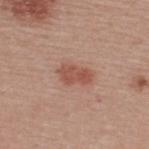Findings:
– biopsy status · total-body-photography surveillance lesion; no biopsy
– location · the upper back
– tile lighting · white-light illumination
– patient · male, about 40 years old
– lesion diameter · ~3.5 mm (longest diameter)
– acquisition · total-body-photography crop, ~15 mm field of view
– TBP lesion metrics · border irregularity of about 3 on a 0–10 scale, a within-lesion color-variation index near 2.5/10, and a peripheral color-asymmetry measure near 1; an automated nevus-likeness rating near 90 out of 100 and a detector confidence of about 100 out of 100 that the crop contains a lesion